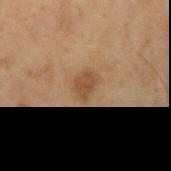{"biopsy_status": "not biopsied; imaged during a skin examination", "image": {"source": "total-body photography crop", "field_of_view_mm": 15}, "lesion_size": {"long_diameter_mm_approx": 3.0}, "patient": {"sex": "male", "age_approx": 70}, "site": "left upper arm"}